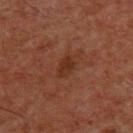  biopsy_status: not biopsied; imaged during a skin examination
  lighting: cross-polarized
  patient:
    sex: male
    age_approx: 60
  site: chest
  automated_metrics:
    cielab_L: 28
    cielab_a: 22
    cielab_b: 28
    vs_skin_darker_L: 7.0
    vs_skin_contrast_norm: 7.0
    border_irregularity_0_10: 3.0
    color_variation_0_10: 1.5
    nevus_likeness_0_100: 0
    lesion_detection_confidence_0_100: 100
  image:
    source: total-body photography crop
    field_of_view_mm: 15